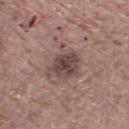Impression: Part of a total-body skin-imaging series; this lesion was reviewed on a skin check and was not flagged for biopsy. Clinical summary: Captured under white-light illumination. The subject is a male aged 68–72. A 15 mm close-up extracted from a 3D total-body photography capture. Automated tile analysis of the lesion measured an eccentricity of roughly 0.6 and a shape-asymmetry score of about 0.35 (0 = symmetric). It also reported a mean CIELAB color near L≈45 a*≈16 b*≈19 and a lesion-to-skin contrast of about 8.5 (normalized; higher = more distinct). The analysis additionally found a border-irregularity rating of about 4.5/10 and internal color variation of about 4 on a 0–10 scale. It also reported a classifier nevus-likeness of about 35/100 and a lesion-detection confidence of about 100/100. The lesion's longest dimension is about 4.5 mm. On the left thigh.